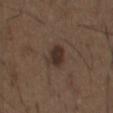Clinical impression:
Captured during whole-body skin photography for melanoma surveillance; the lesion was not biopsied.
Image and clinical context:
Measured at roughly 3 mm in maximum diameter. The lesion is located on the chest. Captured under white-light illumination. Automated image analysis of the tile measured a border-irregularity rating of about 2.5/10 and a peripheral color-asymmetry measure near 1. The analysis additionally found an automated nevus-likeness rating near 85 out of 100 and a detector confidence of about 100 out of 100 that the crop contains a lesion. A male patient, aged approximately 50. A region of skin cropped from a whole-body photographic capture, roughly 15 mm wide.